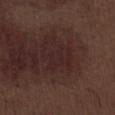This lesion was catalogued during total-body skin photography and was not selected for biopsy.
The lesion's longest dimension is about 4.5 mm.
A 15 mm close-up tile from a total-body photography series done for melanoma screening.
Automated image analysis of the tile measured an area of roughly 9 mm² and an outline eccentricity of about 0.75 (0 = round, 1 = elongated). The software also gave a lesion color around L≈23 a*≈18 b*≈16 in CIELAB, a lesion–skin lightness drop of about 4, and a lesion-to-skin contrast of about 5 (normalized; higher = more distinct). The analysis additionally found a border-irregularity rating of about 7.5/10, a color-variation rating of about 1.5/10, and a peripheral color-asymmetry measure near 0.5. The analysis additionally found an automated nevus-likeness rating near 0 out of 100.
This is a white-light tile.
A male patient, approximately 70 years of age.
The lesion is on the right lower leg.